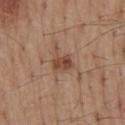<tbp_lesion>
  <biopsy_status>not biopsied; imaged during a skin examination</biopsy_status>
  <lesion_size>
    <long_diameter_mm_approx>3.0</long_diameter_mm_approx>
  </lesion_size>
  <lighting>white-light</lighting>
  <patient>
    <sex>male</sex>
    <age_approx>55</age_approx>
  </patient>
  <image>
    <source>total-body photography crop</source>
    <field_of_view_mm>15</field_of_view_mm>
  </image>
  <site>mid back</site>
</tbp_lesion>